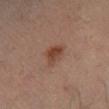A male patient aged around 65. The lesion is on the right lower leg. A 15 mm close-up extracted from a 3D total-body photography capture.